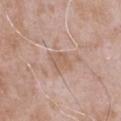Captured during whole-body skin photography for melanoma surveillance; the lesion was not biopsied.
A male subject approximately 50 years of age.
A roughly 15 mm field-of-view crop from a total-body skin photograph.
From the chest.
Approximately 2.5 mm at its widest.
The tile uses white-light illumination.
An algorithmic analysis of the crop reported an area of roughly 4.5 mm², a shape eccentricity near 0.6, and a symmetry-axis asymmetry near 0.2. The analysis additionally found about 7 CIELAB-L* units darker than the surrounding skin and a lesion-to-skin contrast of about 5 (normalized; higher = more distinct). And it measured peripheral color asymmetry of about 0.5.